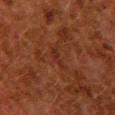follow-up = total-body-photography surveillance lesion; no biopsy | location = the upper back | size = about 3.5 mm | patient = female, aged around 50 | acquisition = ~15 mm crop, total-body skin-cancer survey | tile lighting = cross-polarized | automated metrics = a mean CIELAB color near L≈24 a*≈21 b*≈25, roughly 4 lightness units darker than nearby skin, and a normalized border contrast of about 4.5; internal color variation of about 0.5 on a 0–10 scale and a peripheral color-asymmetry measure near 0; a nevus-likeness score of about 0/100 and lesion-presence confidence of about 80/100.